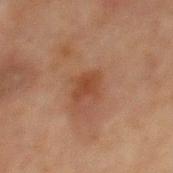Findings:
– biopsy status: imaged on a skin check; not biopsied
– tile lighting: cross-polarized illumination
– site: the abdomen
– size: ≈3 mm
– image-analysis metrics: a footprint of about 5 mm² and a symmetry-axis asymmetry near 0.25; a color-variation rating of about 2/10 and peripheral color asymmetry of about 0.5; a lesion-detection confidence of about 100/100
– imaging modality: total-body-photography crop, ~15 mm field of view
– patient: male, about 65 years old